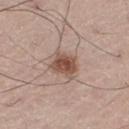follow-up = no biopsy performed (imaged during a skin exam) | site = the abdomen | patient = male, aged 68–72 | lesion diameter = about 3.5 mm | image source = total-body-photography crop, ~15 mm field of view.